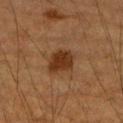Q: Who is the patient?
A: male, about 85 years old or older
Q: How was this image acquired?
A: total-body-photography crop, ~15 mm field of view
Q: What lighting was used for the tile?
A: cross-polarized illumination
Q: Lesion location?
A: the right upper arm
Q: What is the lesion's diameter?
A: about 3.5 mm